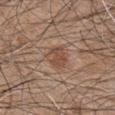{"biopsy_status": "not biopsied; imaged during a skin examination", "image": {"source": "total-body photography crop", "field_of_view_mm": 15}, "patient": {"sex": "male", "age_approx": 45}, "site": "left upper arm"}